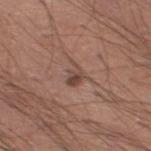Assessment: No biopsy was performed on this lesion — it was imaged during a full skin examination and was not determined to be concerning. Clinical summary: Located on the left lower leg. Approximately 3 mm at its widest. An algorithmic analysis of the crop reported a lesion-to-skin contrast of about 7 (normalized; higher = more distinct). The analysis additionally found a border-irregularity index near 5/10, internal color variation of about 3.5 on a 0–10 scale, and radial color variation of about 1. The analysis additionally found a nevus-likeness score of about 15/100 and a detector confidence of about 100 out of 100 that the crop contains a lesion. Cropped from a total-body skin-imaging series; the visible field is about 15 mm. Captured under white-light illumination. The subject is a male in their 40s.